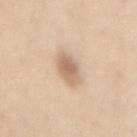{"lighting": "white-light", "patient": {"sex": "female", "age_approx": 30}, "image": {"source": "total-body photography crop", "field_of_view_mm": 15}, "site": "back", "lesion_size": {"long_diameter_mm_approx": 4.0}, "automated_metrics": {"area_mm2_approx": 6.5, "shape_asymmetry": 0.15, "cielab_L": 65, "cielab_a": 17, "cielab_b": 30, "vs_skin_darker_L": 12.0, "vs_skin_contrast_norm": 7.5, "border_irregularity_0_10": 2.0, "color_variation_0_10": 3.5, "peripheral_color_asymmetry": 1.0}}